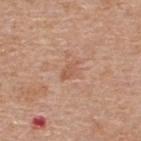<tbp_lesion>
  <patient>
    <sex>male</sex>
    <age_approx>55</age_approx>
  </patient>
  <image>
    <source>total-body photography crop</source>
    <field_of_view_mm>15</field_of_view_mm>
  </image>
  <site>back</site>
</tbp_lesion>